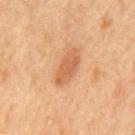This lesion was catalogued during total-body skin photography and was not selected for biopsy.
The lesion is located on the mid back.
A male subject, aged approximately 65.
The recorded lesion diameter is about 4.5 mm.
Imaged with cross-polarized lighting.
Cropped from a whole-body photographic skin survey; the tile spans about 15 mm.
The total-body-photography lesion software estimated a border-irregularity index near 2/10 and a peripheral color-asymmetry measure near 1.